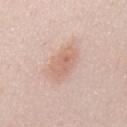Notes:
• follow-up · no biopsy performed (imaged during a skin exam)
• illumination · white-light
• imaging modality · ~15 mm tile from a whole-body skin photo
• subject · male, aged around 25
• size · ≈4.5 mm
• site · the mid back